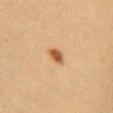<record>
  <biopsy_status>not biopsied; imaged during a skin examination</biopsy_status>
  <lighting>cross-polarized</lighting>
  <image>
    <source>total-body photography crop</source>
    <field_of_view_mm>15</field_of_view_mm>
  </image>
  <site>front of the torso</site>
  <lesion_size>
    <long_diameter_mm_approx>2.5</long_diameter_mm_approx>
  </lesion_size>
  <patient>
    <sex>female</sex>
    <age_approx>40</age_approx>
  </patient>
</record>